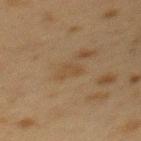Part of a total-body skin-imaging series; this lesion was reviewed on a skin check and was not flagged for biopsy.
The recorded lesion diameter is about 2.5 mm.
A female subject, aged 38 to 42.
An algorithmic analysis of the crop reported a shape eccentricity near 0.75. The analysis additionally found a border-irregularity index near 3/10, a within-lesion color-variation index near 1/10, and a peripheral color-asymmetry measure near 0.5.
Captured under cross-polarized illumination.
Cropped from a whole-body photographic skin survey; the tile spans about 15 mm.
From the mid back.